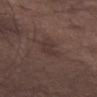<tbp_lesion>
  <biopsy_status>not biopsied; imaged during a skin examination</biopsy_status>
  <site>leg</site>
  <patient>
    <sex>male</sex>
    <age_approx>75</age_approx>
  </patient>
  <image>
    <source>total-body photography crop</source>
    <field_of_view_mm>15</field_of_view_mm>
  </image>
  <automated_metrics>
    <border_irregularity_0_10>4.0</border_irregularity_0_10>
    <color_variation_0_10>1.0</color_variation_0_10>
    <peripheral_color_asymmetry>0.5</peripheral_color_asymmetry>
    <nevus_likeness_0_100>0</nevus_likeness_0_100>
  </automated_metrics>
</tbp_lesion>